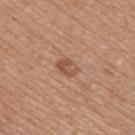Q: What did automated image analysis measure?
A: a mean CIELAB color near L≈52 a*≈22 b*≈31 and a lesion-to-skin contrast of about 6.5 (normalized; higher = more distinct)
Q: What is the lesion's diameter?
A: about 2.5 mm
Q: How was this image acquired?
A: ~15 mm tile from a whole-body skin photo
Q: Where on the body is the lesion?
A: the upper back
Q: What are the patient's age and sex?
A: female, aged 48 to 52